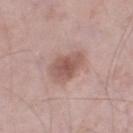Notes:
• biopsy status — catalogued during a skin exam; not biopsied
• subject — male, aged around 55
• body site — the right thigh
• tile lighting — white-light illumination
• image-analysis metrics — a mean CIELAB color near L≈55 a*≈20 b*≈23 and a lesion-to-skin contrast of about 7.5 (normalized; higher = more distinct)
• acquisition — ~15 mm tile from a whole-body skin photo
• lesion diameter — ~4 mm (longest diameter)